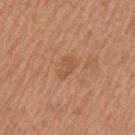follow-up = catalogued during a skin exam; not biopsied
lesion diameter = ≈3 mm
patient = female, aged approximately 50
body site = the left upper arm
automated lesion analysis = a color-variation rating of about 1.5/10 and radial color variation of about 0.5; a detector confidence of about 100 out of 100 that the crop contains a lesion
image source = 15 mm crop, total-body photography